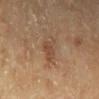<tbp_lesion>
  <biopsy_status>not biopsied; imaged during a skin examination</biopsy_status>
  <site>left lower leg</site>
  <image>
    <source>total-body photography crop</source>
    <field_of_view_mm>15</field_of_view_mm>
  </image>
  <patient>
    <sex>female</sex>
    <age_approx>60</age_approx>
  </patient>
</tbp_lesion>